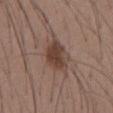Part of a total-body skin-imaging series; this lesion was reviewed on a skin check and was not flagged for biopsy. The subject is a male about 40 years old. A lesion tile, about 15 mm wide, cut from a 3D total-body photograph. The recorded lesion diameter is about 4 mm. Imaged with white-light lighting. Automated image analysis of the tile measured an eccentricity of roughly 0.6. The analysis additionally found roughly 11 lightness units darker than nearby skin and a lesion-to-skin contrast of about 8.5 (normalized; higher = more distinct). It also reported a border-irregularity rating of about 2.5/10, a color-variation rating of about 3/10, and a peripheral color-asymmetry measure near 1. The software also gave lesion-presence confidence of about 100/100. Located on the front of the torso.